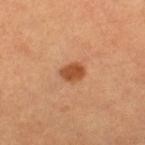Automated image analysis of the tile measured a lesion color around L≈50 a*≈27 b*≈38 in CIELAB, a lesion–skin lightness drop of about 13, and a normalized lesion–skin contrast near 9. It also reported internal color variation of about 2.5 on a 0–10 scale and radial color variation of about 1. The analysis additionally found a detector confidence of about 100 out of 100 that the crop contains a lesion.
A close-up tile cropped from a whole-body skin photograph, about 15 mm across.
The tile uses cross-polarized illumination.
The patient is a female aged approximately 50.